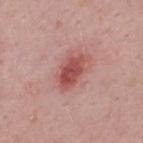biopsy status=catalogued during a skin exam; not biopsied | tile lighting=white-light | site=the upper back | acquisition=~15 mm tile from a whole-body skin photo | diameter=about 4.5 mm | subject=male, approximately 50 years of age.